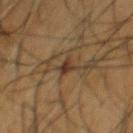Clinical impression: Captured during whole-body skin photography for melanoma surveillance; the lesion was not biopsied.